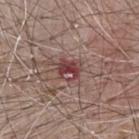Clinical summary:
Imaged with white-light lighting. The recorded lesion diameter is about 2.5 mm. Cropped from a whole-body photographic skin survey; the tile spans about 15 mm. On the chest. The patient is a male aged approximately 65.